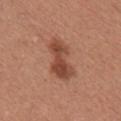<record>
<biopsy_status>not biopsied; imaged during a skin examination</biopsy_status>
<site>right upper arm</site>
<lesion_size>
  <long_diameter_mm_approx>5.5</long_diameter_mm_approx>
</lesion_size>
<lighting>white-light</lighting>
<automated_metrics>
  <eccentricity>0.9</eccentricity>
  <shape_asymmetry>0.3</shape_asymmetry>
  <nevus_likeness_0_100>90</nevus_likeness_0_100>
  <lesion_detection_confidence_0_100>100</lesion_detection_confidence_0_100>
</automated_metrics>
<patient>
  <sex>female</sex>
  <age_approx>25</age_approx>
</patient>
<image>
  <source>total-body photography crop</source>
  <field_of_view_mm>15</field_of_view_mm>
</image>
</record>